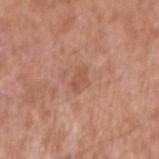<lesion>
  <biopsy_status>not biopsied; imaged during a skin examination</biopsy_status>
  <lesion_size>
    <long_diameter_mm_approx>3.0</long_diameter_mm_approx>
  </lesion_size>
  <site>right upper arm</site>
  <patient>
    <sex>male</sex>
    <age_approx>55</age_approx>
  </patient>
  <image>
    <source>total-body photography crop</source>
    <field_of_view_mm>15</field_of_view_mm>
  </image>
</lesion>